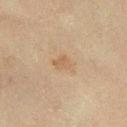Impression: No biopsy was performed on this lesion — it was imaged during a full skin examination and was not determined to be concerning. Clinical summary: The recorded lesion diameter is about 3 mm. The tile uses cross-polarized illumination. A close-up tile cropped from a whole-body skin photograph, about 15 mm across. A male subject approximately 65 years of age. Located on the left lower leg. Automated image analysis of the tile measured a lesion color around L≈48 a*≈13 b*≈28 in CIELAB, roughly 5 lightness units darker than nearby skin, and a lesion-to-skin contrast of about 4.5 (normalized; higher = more distinct). It also reported a border-irregularity rating of about 3/10, internal color variation of about 1.5 on a 0–10 scale, and peripheral color asymmetry of about 0.5. The software also gave an automated nevus-likeness rating near 0 out of 100 and a lesion-detection confidence of about 100/100.